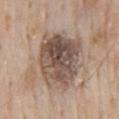biopsy status = no biopsy performed (imaged during a skin exam) | subject = male, aged 58–62 | automated lesion analysis = about 14 CIELAB-L* units darker than the surrounding skin and a normalized lesion–skin contrast near 9.5; internal color variation of about 8.5 on a 0–10 scale and a peripheral color-asymmetry measure near 3; a classifier nevus-likeness of about 25/100 and a detector confidence of about 100 out of 100 that the crop contains a lesion | lighting = white-light illumination | location = the back | image = ~15 mm crop, total-body skin-cancer survey.